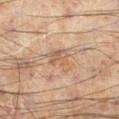<lesion>
<biopsy_status>not biopsied; imaged during a skin examination</biopsy_status>
<image>
  <source>total-body photography crop</source>
  <field_of_view_mm>15</field_of_view_mm>
</image>
<site>left lower leg</site>
<patient>
  <sex>male</sex>
  <age_approx>60</age_approx>
</patient>
</lesion>